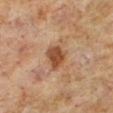Findings:
• workup: no biopsy performed (imaged during a skin exam)